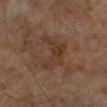{"biopsy_status": "not biopsied; imaged during a skin examination", "lighting": "cross-polarized", "patient": {"sex": "male", "age_approx": 65}, "image": {"source": "total-body photography crop", "field_of_view_mm": 15}, "automated_metrics": {"cielab_L": 34, "cielab_a": 17, "cielab_b": 26, "vs_skin_darker_L": 6.0, "vs_skin_contrast_norm": 6.0, "border_irregularity_0_10": 7.5, "color_variation_0_10": 3.0, "peripheral_color_asymmetry": 1.0, "lesion_detection_confidence_0_100": 100}, "lesion_size": {"long_diameter_mm_approx": 5.0}}